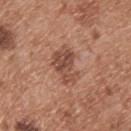Recorded during total-body skin imaging; not selected for excision or biopsy. The lesion is on the back. Automated tile analysis of the lesion measured border irregularity of about 5.5 on a 0–10 scale, a within-lesion color-variation index near 4.5/10, and radial color variation of about 1.5. Captured under white-light illumination. About 4.5 mm across. The patient is a male roughly 55 years of age. A lesion tile, about 15 mm wide, cut from a 3D total-body photograph.